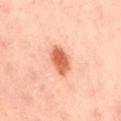| field | value |
|---|---|
| image | total-body-photography crop, ~15 mm field of view |
| image-analysis metrics | an eccentricity of roughly 0.75 and a shape-asymmetry score of about 0.2 (0 = symmetric); a border-irregularity rating of about 2/10, a color-variation rating of about 3.5/10, and a peripheral color-asymmetry measure near 1; a nevus-likeness score of about 100/100 and a detector confidence of about 100 out of 100 that the crop contains a lesion |
| subject | female, about 35 years old |
| location | the lower back |